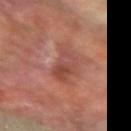No biopsy was performed on this lesion — it was imaged during a full skin examination and was not determined to be concerning.
Automated tile analysis of the lesion measured a nevus-likeness score of about 0/100.
The lesion's longest dimension is about 5.5 mm.
Captured under cross-polarized illumination.
A male subject aged around 70.
The lesion is on the left forearm.
A 15 mm crop from a total-body photograph taken for skin-cancer surveillance.